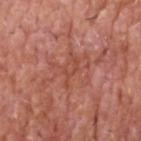Part of a total-body skin-imaging series; this lesion was reviewed on a skin check and was not flagged for biopsy. A male patient, in their 60s. This image is a 15 mm lesion crop taken from a total-body photograph. From the head or neck. The total-body-photography lesion software estimated a shape-asymmetry score of about 0.35 (0 = symmetric). It also reported an average lesion color of about L≈49 a*≈28 b*≈31 (CIELAB), roughly 6 lightness units darker than nearby skin, and a normalized border contrast of about 5. This is a white-light tile. Measured at roughly 3.5 mm in maximum diameter.